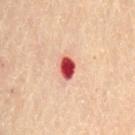Imaged during a routine full-body skin examination; the lesion was not biopsied and no histopathology is available.
The total-body-photography lesion software estimated a symmetry-axis asymmetry near 0.3. The software also gave a border-irregularity rating of about 2.5/10, a color-variation rating of about 3.5/10, and peripheral color asymmetry of about 1.
From the lower back.
Cropped from a whole-body photographic skin survey; the tile spans about 15 mm.
This is a cross-polarized tile.
A female subject aged approximately 60.
The lesion's longest dimension is about 3 mm.